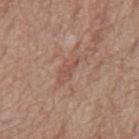{"biopsy_status": "not biopsied; imaged during a skin examination", "lesion_size": {"long_diameter_mm_approx": 2.5}, "site": "back", "automated_metrics": {"cielab_L": 51, "cielab_a": 23, "cielab_b": 26, "vs_skin_darker_L": 6.0, "vs_skin_contrast_norm": 5.0}, "patient": {"sex": "male", "age_approx": 70}, "lighting": "white-light", "image": {"source": "total-body photography crop", "field_of_view_mm": 15}}